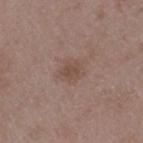Notes:
• biopsy status · no biopsy performed (imaged during a skin exam)
• tile lighting · white-light illumination
• anatomic site · the left thigh
• subject · male, aged 48 to 52
• imaging modality · ~15 mm tile from a whole-body skin photo